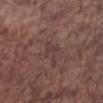Notes:
* follow-up: imaged on a skin check; not biopsied
* diameter: about 3.5 mm
* illumination: white-light
* imaging modality: ~15 mm tile from a whole-body skin photo
* automated metrics: a footprint of about 3.5 mm² and an eccentricity of roughly 0.9; a lesion color around L≈38 a*≈17 b*≈19 in CIELAB, about 5 CIELAB-L* units darker than the surrounding skin, and a normalized lesion–skin contrast near 5; a nevus-likeness score of about 0/100
* location: the right forearm
* patient: male, aged 73 to 77